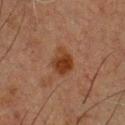  biopsy_status: not biopsied; imaged during a skin examination
  lesion_size:
    long_diameter_mm_approx: 3.5
  automated_metrics:
    area_mm2_approx: 7.0
    eccentricity: 0.65
    shape_asymmetry: 0.2
    border_irregularity_0_10: 2.0
    color_variation_0_10: 4.0
  lighting: cross-polarized
  image:
    source: total-body photography crop
    field_of_view_mm: 15
  site: chest
  patient:
    sex: male
    age_approx: 50